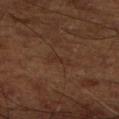follow-up: total-body-photography surveillance lesion; no biopsy
TBP lesion metrics: a shape eccentricity near 0.9 and a shape-asymmetry score of about 0.4 (0 = symmetric); a lesion color around L≈29 a*≈17 b*≈25 in CIELAB, roughly 4 lightness units darker than nearby skin, and a lesion-to-skin contrast of about 4.5 (normalized; higher = more distinct); border irregularity of about 4.5 on a 0–10 scale, a color-variation rating of about 0/10, and a peripheral color-asymmetry measure near 0; a nevus-likeness score of about 0/100 and a lesion-detection confidence of about 95/100
body site: the right lower leg
diameter: ≈2.5 mm
image source: ~15 mm tile from a whole-body skin photo
subject: aged 63–67
illumination: cross-polarized illumination